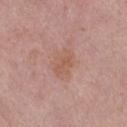Impression:
Recorded during total-body skin imaging; not selected for excision or biopsy.
Image and clinical context:
From the right thigh. A female patient aged around 60. Automated tile analysis of the lesion measured a lesion area of about 7.5 mm², an eccentricity of roughly 0.7, and a shape-asymmetry score of about 0.2 (0 = symmetric). And it measured an average lesion color of about L≈57 a*≈21 b*≈28 (CIELAB) and a lesion–skin lightness drop of about 6. It also reported an automated nevus-likeness rating near 0 out of 100. Imaged with white-light lighting. A 15 mm close-up extracted from a 3D total-body photography capture. The lesion's longest dimension is about 3.5 mm.